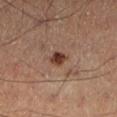| field | value |
|---|---|
| notes | imaged on a skin check; not biopsied |
| diameter | about 2 mm |
| patient | male, aged 48 to 52 |
| imaging modality | total-body-photography crop, ~15 mm field of view |
| anatomic site | the left lower leg |